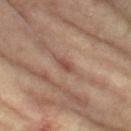{"biopsy_status": "not biopsied; imaged during a skin examination", "lighting": "cross-polarized", "automated_metrics": {"border_irregularity_0_10": 2.5, "color_variation_0_10": 2.5, "peripheral_color_asymmetry": 1.0}, "site": "left thigh", "image": {"source": "total-body photography crop", "field_of_view_mm": 15}, "patient": {"sex": "female", "age_approx": 75}, "lesion_size": {"long_diameter_mm_approx": 2.5}}